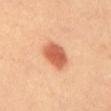This lesion was catalogued during total-body skin photography and was not selected for biopsy.
The total-body-photography lesion software estimated a shape-asymmetry score of about 0.15 (0 = symmetric). And it measured a mean CIELAB color near L≈62 a*≈30 b*≈38 and roughly 15 lightness units darker than nearby skin. It also reported a classifier nevus-likeness of about 100/100 and lesion-presence confidence of about 100/100.
The subject is a female about 30 years old.
Longest diameter approximately 3.5 mm.
The lesion is located on the front of the torso.
A 15 mm close-up extracted from a 3D total-body photography capture.
Captured under cross-polarized illumination.